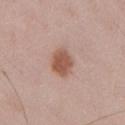{"biopsy_status": "not biopsied; imaged during a skin examination", "patient": {"sex": "male", "age_approx": 55}, "image": {"source": "total-body photography crop", "field_of_view_mm": 15}, "lesion_size": {"long_diameter_mm_approx": 3.5}, "automated_metrics": {"area_mm2_approx": 7.0, "eccentricity": 0.6, "shape_asymmetry": 0.15, "cielab_L": 54, "cielab_a": 22, "cielab_b": 29, "vs_skin_darker_L": 12.0, "nevus_likeness_0_100": 100}, "site": "chest"}